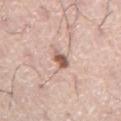Clinical impression:
Captured during whole-body skin photography for melanoma surveillance; the lesion was not biopsied.
Acquisition and patient details:
The lesion is on the abdomen. A male patient aged around 75. A 15 mm crop from a total-body photograph taken for skin-cancer surveillance.